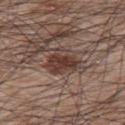Clinical impression:
Recorded during total-body skin imaging; not selected for excision or biopsy.
Context:
A roughly 15 mm field-of-view crop from a total-body skin photograph. Longest diameter approximately 5 mm. A male patient aged 63–67. On the upper back. This is a white-light tile.